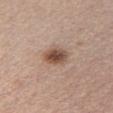Imaged during a routine full-body skin examination; the lesion was not biopsied and no histopathology is available.